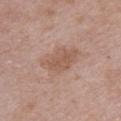<record>
<biopsy_status>not biopsied; imaged during a skin examination</biopsy_status>
</record>